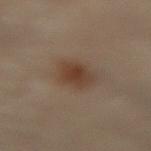Captured during whole-body skin photography for melanoma surveillance; the lesion was not biopsied.
A female patient, aged around 60.
The lesion is located on the front of the torso.
Cropped from a whole-body photographic skin survey; the tile spans about 15 mm.